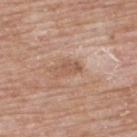Impression:
Imaged during a routine full-body skin examination; the lesion was not biopsied and no histopathology is available.
Acquisition and patient details:
The tile uses white-light illumination. A region of skin cropped from a whole-body photographic capture, roughly 15 mm wide. A male subject, approximately 65 years of age. From the upper back.